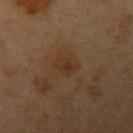Recorded during total-body skin imaging; not selected for excision or biopsy. The lesion-visualizer software estimated a lesion area of about 4 mm², an eccentricity of roughly 0.75, and a shape-asymmetry score of about 0.25 (0 = symmetric). The software also gave a border-irregularity index near 2.5/10 and peripheral color asymmetry of about 0.5. Approximately 2.5 mm at its widest. From the left upper arm. A female subject, aged approximately 40. Imaged with cross-polarized lighting. A 15 mm close-up extracted from a 3D total-body photography capture.